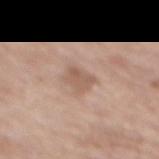Q: Is there a histopathology result?
A: imaged on a skin check; not biopsied
Q: Patient demographics?
A: male, in their 70s
Q: What kind of image is this?
A: ~15 mm tile from a whole-body skin photo
Q: Automated lesion metrics?
A: a lesion area of about 5 mm², an eccentricity of roughly 0.65, and a shape-asymmetry score of about 0.2 (0 = symmetric)
Q: Lesion size?
A: ≈2.5 mm
Q: What is the anatomic site?
A: the mid back
Q: How was the tile lit?
A: white-light illumination